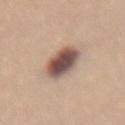| feature | finding |
|---|---|
| follow-up | total-body-photography surveillance lesion; no biopsy |
| TBP lesion metrics | a border-irregularity index near 1/10, internal color variation of about 8 on a 0–10 scale, and a peripheral color-asymmetry measure near 2 |
| image source | ~15 mm crop, total-body skin-cancer survey |
| anatomic site | the lower back |
| diameter | ~4.5 mm (longest diameter) |
| patient | female, roughly 25 years of age |
| tile lighting | white-light illumination |